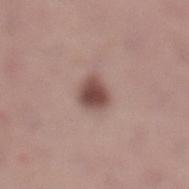{
  "patient": {
    "sex": "female",
    "age_approx": 40
  },
  "image": {
    "source": "total-body photography crop",
    "field_of_view_mm": 15
  },
  "site": "leg"
}